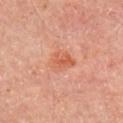Clinical impression: Recorded during total-body skin imaging; not selected for excision or biopsy. Acquisition and patient details: About 3.5 mm across. The lesion is on the arm. The lesion-visualizer software estimated an outline eccentricity of about 0.65 (0 = round, 1 = elongated) and a shape-asymmetry score of about 0.25 (0 = symmetric). And it measured an average lesion color of about L≈58 a*≈29 b*≈35 (CIELAB), roughly 8 lightness units darker than nearby skin, and a normalized lesion–skin contrast near 6. And it measured a border-irregularity index near 2.5/10, a color-variation rating of about 4/10, and radial color variation of about 1. The patient is a male aged around 65. A 15 mm close-up extracted from a 3D total-body photography capture.